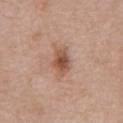Assessment:
Part of a total-body skin-imaging series; this lesion was reviewed on a skin check and was not flagged for biopsy.
Clinical summary:
A male subject in their mid-70s. A 15 mm close-up tile from a total-body photography series done for melanoma screening. Approximately 3.5 mm at its widest. The lesion is located on the abdomen. The total-body-photography lesion software estimated an outline eccentricity of about 0.7 (0 = round, 1 = elongated) and a shape-asymmetry score of about 0.2 (0 = symmetric). It also reported border irregularity of about 2 on a 0–10 scale, a within-lesion color-variation index near 4.5/10, and peripheral color asymmetry of about 1. And it measured a nevus-likeness score of about 85/100 and a detector confidence of about 100 out of 100 that the crop contains a lesion. This is a white-light tile.